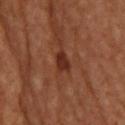About 2.5 mm across. From the upper back. A roughly 15 mm field-of-view crop from a total-body skin photograph. Automated tile analysis of the lesion measured an outline eccentricity of about 0.75 (0 = round, 1 = elongated) and a shape-asymmetry score of about 0.25 (0 = symmetric). It also reported a border-irregularity index near 2.5/10 and peripheral color asymmetry of about 0.5. It also reported a classifier nevus-likeness of about 45/100 and a lesion-detection confidence of about 100/100. The tile uses cross-polarized illumination.